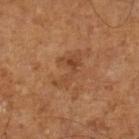biopsy status: catalogued during a skin exam; not biopsied